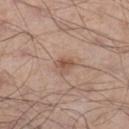Notes:
* biopsy status · catalogued during a skin exam; not biopsied
* subject · male, aged approximately 55
* lesion diameter · ≈2.5 mm
* lighting · white-light
* TBP lesion metrics · a mean CIELAB color near L≈52 a*≈19 b*≈28 and a lesion–skin lightness drop of about 10; border irregularity of about 3.5 on a 0–10 scale, a color-variation rating of about 2/10, and a peripheral color-asymmetry measure near 1; an automated nevus-likeness rating near 60 out of 100 and a detector confidence of about 100 out of 100 that the crop contains a lesion
* body site · the leg
* image · 15 mm crop, total-body photography